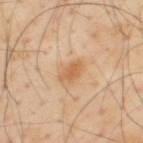The lesion was tiled from a total-body skin photograph and was not biopsied.
The lesion's longest dimension is about 3 mm.
Automated tile analysis of the lesion measured a footprint of about 4 mm², an eccentricity of roughly 0.85, and a symmetry-axis asymmetry near 0.3. The analysis additionally found a classifier nevus-likeness of about 75/100.
A 15 mm crop from a total-body photograph taken for skin-cancer surveillance.
Imaged with cross-polarized lighting.
A male patient aged approximately 55.
On the upper back.